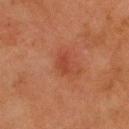The lesion was photographed on a routine skin check and not biopsied; there is no pathology result.
On the head or neck.
Imaged with cross-polarized lighting.
Cropped from a whole-body photographic skin survey; the tile spans about 15 mm.
An algorithmic analysis of the crop reported an area of roughly 3 mm² and a shape eccentricity near 0.85.
A male patient in their mid- to late 50s.